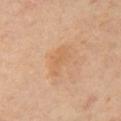Case summary:
• follow-up — catalogued during a skin exam; not biopsied
• subject — female, in their mid- to late 40s
• body site — the right upper arm
• image-analysis metrics — an area of roughly 7 mm² and two-axis asymmetry of about 0.35; roughly 6 lightness units darker than nearby skin and a normalized border contrast of about 4.5; a border-irregularity rating of about 4.5/10 and radial color variation of about 0.5
• tile lighting — cross-polarized
• image — ~15 mm crop, total-body skin-cancer survey
• lesion size — ≈4.5 mm